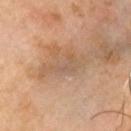notes — no biopsy performed (imaged during a skin exam)
anatomic site — the head or neck
subject — male, in their 60s
lighting — cross-polarized
automated metrics — a lesion–skin lightness drop of about 7; a within-lesion color-variation index near 3.5/10 and a peripheral color-asymmetry measure near 1; an automated nevus-likeness rating near 0 out of 100 and a lesion-detection confidence of about 100/100
image — total-body-photography crop, ~15 mm field of view
lesion size — ~6 mm (longest diameter)